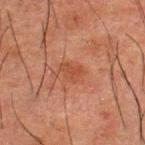Recorded during total-body skin imaging; not selected for excision or biopsy.
Automated tile analysis of the lesion measured a shape eccentricity near 0.7 and a shape-asymmetry score of about 0.25 (0 = symmetric). The analysis additionally found a lesion color around L≈38 a*≈22 b*≈28 in CIELAB, about 5 CIELAB-L* units darker than the surrounding skin, and a normalized border contrast of about 5.5. It also reported a color-variation rating of about 1.5/10 and a peripheral color-asymmetry measure near 0.5. It also reported lesion-presence confidence of about 100/100.
On the upper back.
A male patient, aged 48–52.
About 3 mm across.
A 15 mm close-up tile from a total-body photography series done for melanoma screening.